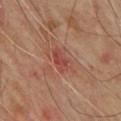Q: Was this lesion biopsied?
A: imaged on a skin check; not biopsied
Q: What is the imaging modality?
A: ~15 mm crop, total-body skin-cancer survey
Q: Patient demographics?
A: male, roughly 65 years of age
Q: Lesion location?
A: the upper back